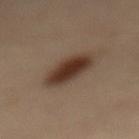Approximately 6 mm at its widest.
An algorithmic analysis of the crop reported an area of roughly 13 mm² and a shape eccentricity near 0.85. It also reported a detector confidence of about 100 out of 100 that the crop contains a lesion.
This is a cross-polarized tile.
A region of skin cropped from a whole-body photographic capture, roughly 15 mm wide.
The subject is a female aged 63 to 67.
On the mid back.